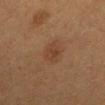workup = catalogued during a skin exam; not biopsied
anatomic site = the leg
lesion diameter = about 3.5 mm
patient = female, in their 40s
image = total-body-photography crop, ~15 mm field of view